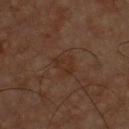Recorded during total-body skin imaging; not selected for excision or biopsy.
A region of skin cropped from a whole-body photographic capture, roughly 15 mm wide.
A male subject aged 58 to 62.
The lesion is located on the chest.